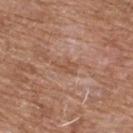Case summary:
- biopsy status · total-body-photography surveillance lesion; no biopsy
- automated lesion analysis · a shape eccentricity near 0.8; a nevus-likeness score of about 0/100 and a detector confidence of about 95 out of 100 that the crop contains a lesion
- tile lighting · white-light
- body site · the chest
- patient · male, aged 58 to 62
- acquisition · ~15 mm crop, total-body skin-cancer survey